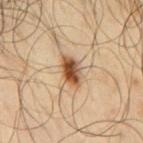The lesion was photographed on a routine skin check and not biopsied; there is no pathology result. A male patient aged around 65. Cropped from a whole-body photographic skin survey; the tile spans about 15 mm. Located on the back. Measured at roughly 4 mm in maximum diameter. Imaged with cross-polarized lighting.